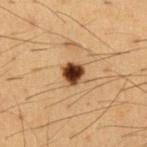workup: no biopsy performed (imaged during a skin exam) | anatomic site: the chest | subject: male, aged 48–52 | TBP lesion metrics: a lesion area of about 5.5 mm², an outline eccentricity of about 0.35 (0 = round, 1 = elongated), and a shape-asymmetry score of about 0.2 (0 = symmetric); an average lesion color of about L≈32 a*≈18 b*≈28 (CIELAB), a lesion–skin lightness drop of about 19, and a lesion-to-skin contrast of about 16 (normalized; higher = more distinct); a within-lesion color-variation index near 5/10 and peripheral color asymmetry of about 1.5; a nevus-likeness score of about 100/100 and lesion-presence confidence of about 100/100 | tile lighting: cross-polarized illumination | lesion size: about 2.5 mm | acquisition: ~15 mm crop, total-body skin-cancer survey.